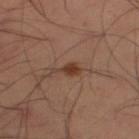– biopsy status: imaged on a skin check; not biopsied
– size: ≈3 mm
– tile lighting: cross-polarized illumination
– patient: male, in their mid- to late 30s
– image: ~15 mm tile from a whole-body skin photo
– body site: the right thigh